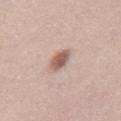automated lesion analysis — a lesion area of about 5 mm² and two-axis asymmetry of about 0.2; a border-irregularity index near 2/10 and radial color variation of about 1 | lesion diameter — about 3 mm | image — total-body-photography crop, ~15 mm field of view | site — the front of the torso | subject — male, aged approximately 45.